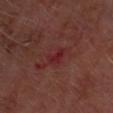<case>
  <biopsy_status>not biopsied; imaged during a skin examination</biopsy_status>
  <patient>
    <sex>male</sex>
    <age_approx>60</age_approx>
  </patient>
  <image>
    <source>total-body photography crop</source>
    <field_of_view_mm>15</field_of_view_mm>
  </image>
  <lesion_size>
    <long_diameter_mm_approx>2.5</long_diameter_mm_approx>
  </lesion_size>
  <site>head or neck</site>
  <lighting>cross-polarized</lighting>
  <automated_metrics>
    <area_mm2_approx>3.0</area_mm2_approx>
    <eccentricity>0.85</eccentricity>
    <shape_asymmetry>0.4</shape_asymmetry>
    <cielab_L>25</cielab_L>
    <cielab_a>28</cielab_a>
    <cielab_b>20</cielab_b>
    <vs_skin_darker_L>6.0</vs_skin_darker_L>
    <vs_skin_contrast_norm>6.0</vs_skin_contrast_norm>
    <nevus_likeness_0_100>0</nevus_likeness_0_100>
  </automated_metrics>
</case>